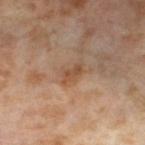Assessment:
Part of a total-body skin-imaging series; this lesion was reviewed on a skin check and was not flagged for biopsy.
Background:
A region of skin cropped from a whole-body photographic capture, roughly 15 mm wide. The patient is a female in their mid- to late 50s. From the left thigh.